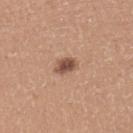{"biopsy_status": "not biopsied; imaged during a skin examination", "patient": {"sex": "female", "age_approx": 35}, "lighting": "white-light", "image": {"source": "total-body photography crop", "field_of_view_mm": 15}, "site": "left lower leg"}